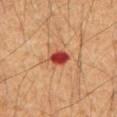<case>
  <biopsy_status>not biopsied; imaged during a skin examination</biopsy_status>
  <image>
    <source>total-body photography crop</source>
    <field_of_view_mm>15</field_of_view_mm>
  </image>
  <patient>
    <sex>male</sex>
    <age_approx>60</age_approx>
  </patient>
  <lesion_size>
    <long_diameter_mm_approx>3.0</long_diameter_mm_approx>
  </lesion_size>
  <lighting>cross-polarized</lighting>
  <site>back</site>
</case>